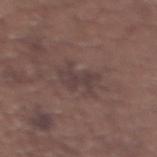Assessment:
This lesion was catalogued during total-body skin photography and was not selected for biopsy.
Context:
A female subject, in their mid- to late 60s. From the upper back. A 15 mm close-up extracted from a 3D total-body photography capture.